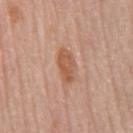Assessment: Recorded during total-body skin imaging; not selected for excision or biopsy. Image and clinical context: A male patient, aged 78–82. Automated image analysis of the tile measured a lesion area of about 7.5 mm² and a symmetry-axis asymmetry near 0.2. It also reported a lesion color around L≈56 a*≈23 b*≈33 in CIELAB. The software also gave border irregularity of about 2.5 on a 0–10 scale, a color-variation rating of about 3/10, and radial color variation of about 1. From the chest. A 15 mm crop from a total-body photograph taken for skin-cancer surveillance.